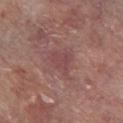{
  "biopsy_status": "not biopsied; imaged during a skin examination",
  "image": {
    "source": "total-body photography crop",
    "field_of_view_mm": 15
  },
  "lighting": "white-light",
  "patient": {
    "sex": "male",
    "age_approx": 70
  },
  "lesion_size": {
    "long_diameter_mm_approx": 3.0
  },
  "site": "right lower leg"
}